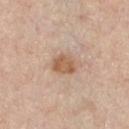workup = imaged on a skin check; not biopsied
diameter = ~3 mm (longest diameter)
location = the chest
illumination = white-light illumination
acquisition = 15 mm crop, total-body photography
subject = male, aged 58–62